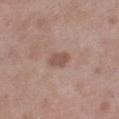Findings:
- biopsy status: total-body-photography surveillance lesion; no biopsy
- image: ~15 mm tile from a whole-body skin photo
- subject: male, aged approximately 70
- diameter: ≈3 mm
- site: the right lower leg
- tile lighting: white-light illumination